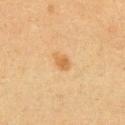follow-up: total-body-photography surveillance lesion; no biopsy | tile lighting: cross-polarized illumination | subject: male, aged 53 to 57 | imaging modality: ~15 mm tile from a whole-body skin photo | lesion diameter: ~2.5 mm (longest diameter) | location: the abdomen.